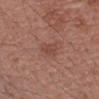<tbp_lesion>
  <patient>
    <sex>female</sex>
    <age_approx>55</age_approx>
  </patient>
  <image>
    <source>total-body photography crop</source>
    <field_of_view_mm>15</field_of_view_mm>
  </image>
  <lesion_size>
    <long_diameter_mm_approx>2.5</long_diameter_mm_approx>
  </lesion_size>
  <site>arm</site>
</tbp_lesion>